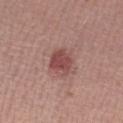No biopsy was performed on this lesion — it was imaged during a full skin examination and was not determined to be concerning. A male subject approximately 30 years of age. Cropped from a whole-body photographic skin survey; the tile spans about 15 mm. Captured under white-light illumination.